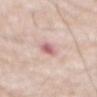Clinical impression: Recorded during total-body skin imaging; not selected for excision or biopsy. Acquisition and patient details: The lesion is located on the abdomen. A male subject aged around 80. A 15 mm close-up tile from a total-body photography series done for melanoma screening. An algorithmic analysis of the crop reported a lesion area of about 3.5 mm² and a symmetry-axis asymmetry near 0.2. Measured at roughly 3 mm in maximum diameter. Imaged with white-light lighting.